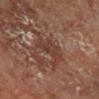- workup — no biopsy performed (imaged during a skin exam)
- tile lighting — cross-polarized
- diameter — ≈4 mm
- patient — male, roughly 65 years of age
- body site — the right lower leg
- imaging modality — total-body-photography crop, ~15 mm field of view